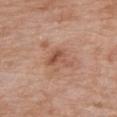{"biopsy_status": "not biopsied; imaged during a skin examination", "site": "chest", "lesion_size": {"long_diameter_mm_approx": 4.0}, "patient": {"sex": "male", "age_approx": 80}, "image": {"source": "total-body photography crop", "field_of_view_mm": 15}}